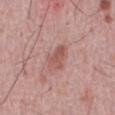{
  "biopsy_status": "not biopsied; imaged during a skin examination",
  "patient": {
    "sex": "male",
    "age_approx": 50
  },
  "image": {
    "source": "total-body photography crop",
    "field_of_view_mm": 15
  },
  "site": "front of the torso",
  "automated_metrics": {
    "eccentricity": 0.75,
    "shape_asymmetry": 0.4,
    "border_irregularity_0_10": 4.0,
    "peripheral_color_asymmetry": 1.0,
    "nevus_likeness_0_100": 20,
    "lesion_detection_confidence_0_100": 100
  }
}